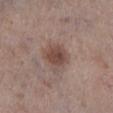The lesion was photographed on a routine skin check and not biopsied; there is no pathology result. The recorded lesion diameter is about 3.5 mm. Imaged with white-light lighting. From the left lower leg. A lesion tile, about 15 mm wide, cut from a 3D total-body photograph. A male subject in their mid-60s.